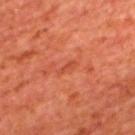Notes:
* biopsy status · imaged on a skin check; not biopsied
* subject · male, approximately 65 years of age
* imaging modality · 15 mm crop, total-body photography
* body site · the upper back